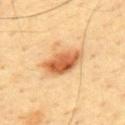No biopsy was performed on this lesion — it was imaged during a full skin examination and was not determined to be concerning.
The lesion is on the back.
A male patient approximately 45 years of age.
The tile uses cross-polarized illumination.
Measured at roughly 5 mm in maximum diameter.
A roughly 15 mm field-of-view crop from a total-body skin photograph.